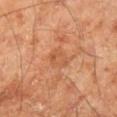The patient is a male aged 68 to 72. The lesion-visualizer software estimated an area of roughly 5 mm², an eccentricity of roughly 0.75, and a shape-asymmetry score of about 0.25 (0 = symmetric). The software also gave an average lesion color of about L≈54 a*≈26 b*≈38 (CIELAB), roughly 6 lightness units darker than nearby skin, and a lesion-to-skin contrast of about 5 (normalized; higher = more distinct). It also reported internal color variation of about 3.5 on a 0–10 scale and peripheral color asymmetry of about 1.5. It also reported a nevus-likeness score of about 0/100. A 15 mm close-up tile from a total-body photography series done for melanoma screening. The recorded lesion diameter is about 3 mm. The lesion is located on the right lower leg. Captured under cross-polarized illumination.